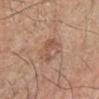notes: no biopsy performed (imaged during a skin exam)
TBP lesion metrics: a lesion area of about 5 mm² and an outline eccentricity of about 0.8 (0 = round, 1 = elongated)
illumination: cross-polarized
lesion size: about 3.5 mm
patient: in their mid-60s
image: total-body-photography crop, ~15 mm field of view
location: the right lower leg